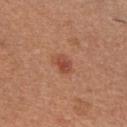biopsy_status: not biopsied; imaged during a skin examination
patient:
  sex: male
  age_approx: 40
lesion_size:
  long_diameter_mm_approx: 3.0
image:
  source: total-body photography crop
  field_of_view_mm: 15
automated_metrics:
  area_mm2_approx: 4.5
  eccentricity: 0.75
  shape_asymmetry: 0.25
  border_irregularity_0_10: 2.5
  color_variation_0_10: 3.5
  peripheral_color_asymmetry: 1.0
  nevus_likeness_0_100: 60
site: chest
lighting: white-light